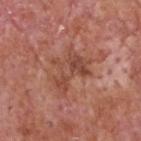Captured during whole-body skin photography for melanoma surveillance; the lesion was not biopsied. An algorithmic analysis of the crop reported an area of roughly 11 mm², an outline eccentricity of about 0.75 (0 = round, 1 = elongated), and two-axis asymmetry of about 0.55. The analysis additionally found a mean CIELAB color near L≈47 a*≈25 b*≈29, roughly 8 lightness units darker than nearby skin, and a normalized border contrast of about 6.5. The analysis additionally found border irregularity of about 8.5 on a 0–10 scale and a peripheral color-asymmetry measure near 1.5. It also reported a classifier nevus-likeness of about 0/100 and a detector confidence of about 100 out of 100 that the crop contains a lesion. This image is a 15 mm lesion crop taken from a total-body photograph. The lesion's longest dimension is about 5 mm. Imaged with white-light lighting. On the head or neck. A male patient aged approximately 60.